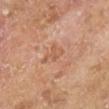| feature | finding |
|---|---|
| follow-up | total-body-photography surveillance lesion; no biopsy |
| imaging modality | total-body-photography crop, ~15 mm field of view |
| anatomic site | the right lower leg |
| subject | male, about 65 years old |
| lesion diameter | ~3 mm (longest diameter) |